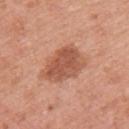<tbp_lesion>
  <biopsy_status>not biopsied; imaged during a skin examination</biopsy_status>
  <patient>
    <sex>female</sex>
    <age_approx>50</age_approx>
  </patient>
  <automated_metrics>
    <cielab_L>55</cielab_L>
    <cielab_a>25</cielab_a>
    <cielab_b>32</cielab_b>
    <vs_skin_darker_L>11.0</vs_skin_darker_L>
    <vs_skin_contrast_norm>7.5</vs_skin_contrast_norm>
  </automated_metrics>
  <site>left upper arm</site>
  <image>
    <source>total-body photography crop</source>
    <field_of_view_mm>15</field_of_view_mm>
  </image>
  <lesion_size>
    <long_diameter_mm_approx>5.5</long_diameter_mm_approx>
  </lesion_size>
  <lighting>white-light</lighting>
</tbp_lesion>